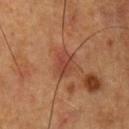Captured during whole-body skin photography for melanoma surveillance; the lesion was not biopsied. A region of skin cropped from a whole-body photographic capture, roughly 15 mm wide. This is a cross-polarized tile. The lesion's longest dimension is about 3 mm. From the front of the torso. The subject is a male roughly 55 years of age. The lesion-visualizer software estimated a footprint of about 5 mm² and two-axis asymmetry of about 0.2. And it measured a nevus-likeness score of about 0/100 and a lesion-detection confidence of about 100/100.